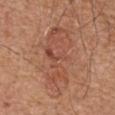Impression: The lesion was photographed on a routine skin check and not biopsied; there is no pathology result. Clinical summary: The lesion-visualizer software estimated about 7 CIELAB-L* units darker than the surrounding skin and a lesion-to-skin contrast of about 5 (normalized; higher = more distinct). And it measured border irregularity of about 5.5 on a 0–10 scale, a color-variation rating of about 5.5/10, and a peripheral color-asymmetry measure near 2. The lesion is located on the chest. Approximately 9 mm at its widest. Cropped from a whole-body photographic skin survey; the tile spans about 15 mm. A male subject roughly 65 years of age. Imaged with white-light lighting.